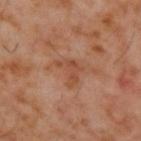notes = total-body-photography surveillance lesion; no biopsy | lighting = cross-polarized illumination | lesion diameter = ≈3.5 mm | subject = male, roughly 60 years of age | location = the upper back | image source = 15 mm crop, total-body photography.